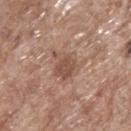Clinical impression:
Recorded during total-body skin imaging; not selected for excision or biopsy.
Image and clinical context:
The lesion is on the upper back. A lesion tile, about 15 mm wide, cut from a 3D total-body photograph. The total-body-photography lesion software estimated a lesion area of about 8 mm² and an eccentricity of roughly 0.7. The analysis additionally found an automated nevus-likeness rating near 0 out of 100. About 4 mm across. A male patient, aged 68–72. This is a white-light tile.